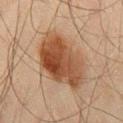This lesion was catalogued during total-body skin photography and was not selected for biopsy. The total-body-photography lesion software estimated a footprint of about 27 mm² and an outline eccentricity of about 0.75 (0 = round, 1 = elongated). It also reported a lesion color around L≈39 a*≈19 b*≈28 in CIELAB, roughly 12 lightness units darker than nearby skin, and a normalized lesion–skin contrast near 10. The analysis additionally found internal color variation of about 7 on a 0–10 scale and a peripheral color-asymmetry measure near 2. A 15 mm close-up tile from a total-body photography series done for melanoma screening. The patient is a male roughly 45 years of age. The tile uses cross-polarized illumination. From the right thigh.